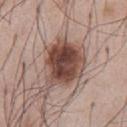Q: Lesion size?
A: ≈6.5 mm
Q: What kind of image is this?
A: 15 mm crop, total-body photography
Q: Illumination type?
A: white-light
Q: Patient demographics?
A: male, in their mid- to late 50s
Q: Where on the body is the lesion?
A: the front of the torso